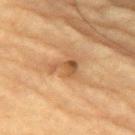Q: Was this lesion biopsied?
A: total-body-photography surveillance lesion; no biopsy
Q: What is the imaging modality?
A: total-body-photography crop, ~15 mm field of view
Q: What are the patient's age and sex?
A: male, aged approximately 85 or older
Q: Where on the body is the lesion?
A: the chest